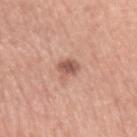<lesion>
  <biopsy_status>not biopsied; imaged during a skin examination</biopsy_status>
  <patient>
    <sex>male</sex>
    <age_approx>60</age_approx>
  </patient>
  <lesion_size>
    <long_diameter_mm_approx>3.0</long_diameter_mm_approx>
  </lesion_size>
  <lighting>white-light</lighting>
  <site>left upper arm</site>
  <image>
    <source>total-body photography crop</source>
    <field_of_view_mm>15</field_of_view_mm>
  </image>
</lesion>